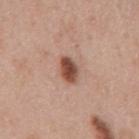| feature | finding |
|---|---|
| notes | total-body-photography surveillance lesion; no biopsy |
| image | ~15 mm crop, total-body skin-cancer survey |
| diameter | about 3.5 mm |
| subject | male, in their 60s |
| image-analysis metrics | a footprint of about 5.5 mm² and a shape-asymmetry score of about 0.2 (0 = symmetric); a lesion color around L≈48 a*≈22 b*≈28 in CIELAB and a lesion–skin lightness drop of about 16; a nevus-likeness score of about 100/100 and a detector confidence of about 100 out of 100 that the crop contains a lesion |
| anatomic site | the mid back |
| illumination | white-light illumination |